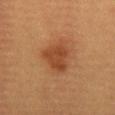Assessment: Part of a total-body skin-imaging series; this lesion was reviewed on a skin check and was not flagged for biopsy. Clinical summary: Located on the front of the torso. Automated tile analysis of the lesion measured an average lesion color of about L≈41 a*≈24 b*≈34 (CIELAB), a lesion–skin lightness drop of about 9, and a lesion-to-skin contrast of about 7.5 (normalized; higher = more distinct). The lesion's longest dimension is about 4 mm. A female patient about 30 years old. A 15 mm close-up tile from a total-body photography series done for melanoma screening.